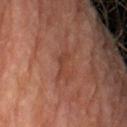Captured during whole-body skin photography for melanoma surveillance; the lesion was not biopsied.
This image is a 15 mm lesion crop taken from a total-body photograph.
The tile uses cross-polarized illumination.
Approximately 4 mm at its widest.
A male patient in their mid- to late 70s.
From the left upper arm.